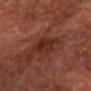Assessment: Part of a total-body skin-imaging series; this lesion was reviewed on a skin check and was not flagged for biopsy. Context: Located on the head or neck. The tile uses cross-polarized illumination. A female patient, roughly 55 years of age. The total-body-photography lesion software estimated a footprint of about 8.5 mm². Cropped from a whole-body photographic skin survey; the tile spans about 15 mm. Longest diameter approximately 4 mm.